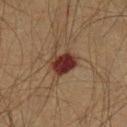Q: Was a biopsy performed?
A: no biopsy performed (imaged during a skin exam)
Q: Lesion location?
A: the left lower leg
Q: What kind of image is this?
A: total-body-photography crop, ~15 mm field of view
Q: Who is the patient?
A: male, roughly 50 years of age
Q: How was the tile lit?
A: cross-polarized illumination